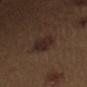acquisition: ~15 mm tile from a whole-body skin photo | site: the right upper arm | lighting: white-light | diameter: ≈3.5 mm | patient: male, about 70 years old.